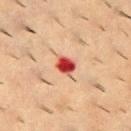Q: What is the imaging modality?
A: 15 mm crop, total-body photography
Q: What is the lesion's diameter?
A: about 2.5 mm
Q: Where on the body is the lesion?
A: the upper back
Q: What are the patient's age and sex?
A: male, approximately 55 years of age
Q: Automated lesion metrics?
A: an average lesion color of about L≈45 a*≈36 b*≈31 (CIELAB) and roughly 18 lightness units darker than nearby skin; a border-irregularity rating of about 2/10 and a color-variation rating of about 4/10
Q: How was the tile lit?
A: cross-polarized